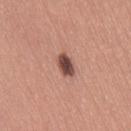The lesion was photographed on a routine skin check and not biopsied; there is no pathology result. This is a white-light tile. Automated image analysis of the tile measured a footprint of about 4.5 mm² and a symmetry-axis asymmetry near 0.25. The analysis additionally found a nevus-likeness score of about 90/100 and lesion-presence confidence of about 100/100. A close-up tile cropped from a whole-body skin photograph, about 15 mm across. A female patient, approximately 25 years of age. From the right thigh.